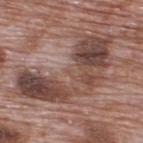The lesion was tiled from a total-body skin photograph and was not biopsied. A male subject about 70 years old. The lesion is on the upper back. The tile uses white-light illumination. Longest diameter approximately 11.5 mm. A roughly 15 mm field-of-view crop from a total-body skin photograph.